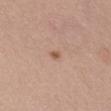Captured during whole-body skin photography for melanoma surveillance; the lesion was not biopsied.
The lesion is located on the head or neck.
A female patient about 25 years old.
The lesion's longest dimension is about 1.5 mm.
Imaged with white-light lighting.
A region of skin cropped from a whole-body photographic capture, roughly 15 mm wide.
Automated image analysis of the tile measured a shape eccentricity near 0.55 and a symmetry-axis asymmetry near 0.25. The analysis additionally found a border-irregularity index near 2/10, a within-lesion color-variation index near 0/10, and peripheral color asymmetry of about 0. And it measured an automated nevus-likeness rating near 55 out of 100 and a lesion-detection confidence of about 100/100.